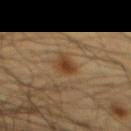Case summary:
– notes: total-body-photography surveillance lesion; no biopsy
– image: total-body-photography crop, ~15 mm field of view
– subject: male, aged approximately 60
– body site: the abdomen